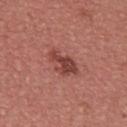{
  "biopsy_status": "not biopsied; imaged during a skin examination",
  "site": "back",
  "lesion_size": {
    "long_diameter_mm_approx": 4.0
  },
  "patient": {
    "sex": "male",
    "age_approx": 40
  },
  "image": {
    "source": "total-body photography crop",
    "field_of_view_mm": 15
  }
}